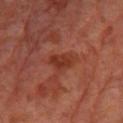Recorded during total-body skin imaging; not selected for excision or biopsy. A 15 mm crop from a total-body photograph taken for skin-cancer surveillance. A male subject, aged approximately 70. On the leg.